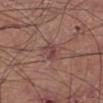notes = catalogued during a skin exam; not biopsied | subject = male, in their mid-40s | body site = the left lower leg | diameter = about 3 mm | image source = total-body-photography crop, ~15 mm field of view | tile lighting = white-light illumination.